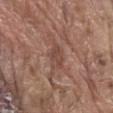Assessment: Captured during whole-body skin photography for melanoma surveillance; the lesion was not biopsied. Background: The patient is a male aged approximately 80. A 15 mm close-up tile from a total-body photography series done for melanoma screening. Automated image analysis of the tile measured a border-irregularity rating of about 2/10, a within-lesion color-variation index near 2.5/10, and a peripheral color-asymmetry measure near 0.5. Measured at roughly 3.5 mm in maximum diameter. The lesion is located on the abdomen. The tile uses white-light illumination.